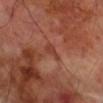The lesion was photographed on a routine skin check and not biopsied; there is no pathology result.
Imaged with cross-polarized lighting.
A close-up tile cropped from a whole-body skin photograph, about 15 mm across.
The lesion is located on the right upper arm.
A male subject, roughly 70 years of age.